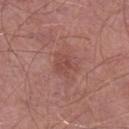Clinical impression: No biopsy was performed on this lesion — it was imaged during a full skin examination and was not determined to be concerning. Background: About 3 mm across. Cropped from a whole-body photographic skin survey; the tile spans about 15 mm. Captured under white-light illumination. Located on the right lower leg. The patient is a male aged 53–57. Automated tile analysis of the lesion measured a lesion area of about 5.5 mm² and two-axis asymmetry of about 0.4. It also reported a border-irregularity rating of about 4/10, a within-lesion color-variation index near 2.5/10, and a peripheral color-asymmetry measure near 1. The software also gave a nevus-likeness score of about 0/100 and lesion-presence confidence of about 100/100.